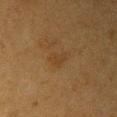Recorded during total-body skin imaging; not selected for excision or biopsy. A 15 mm close-up tile from a total-body photography series done for melanoma screening. The patient is a female about 40 years old. The lesion is on the right upper arm. Captured under cross-polarized illumination. An algorithmic analysis of the crop reported a lesion area of about 3.5 mm², an eccentricity of roughly 0.8, and two-axis asymmetry of about 0.35.